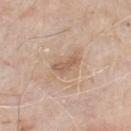The lesion was tiled from a total-body skin photograph and was not biopsied. Automated image analysis of the tile measured a lesion color around L≈58 a*≈18 b*≈30 in CIELAB, about 9 CIELAB-L* units darker than the surrounding skin, and a normalized lesion–skin contrast near 6.5. Longest diameter approximately 3 mm. The tile uses white-light illumination. A 15 mm close-up extracted from a 3D total-body photography capture. The lesion is on the front of the torso. The patient is a male roughly 80 years of age.